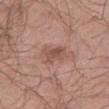The lesion was photographed on a routine skin check and not biopsied; there is no pathology result. A male patient aged 38–42. The tile uses white-light illumination. Measured at roughly 3 mm in maximum diameter. This image is a 15 mm lesion crop taken from a total-body photograph. The lesion is on the right thigh.